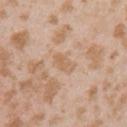<tbp_lesion>
  <biopsy_status>not biopsied; imaged during a skin examination</biopsy_status>
  <image>
    <source>total-body photography crop</source>
    <field_of_view_mm>15</field_of_view_mm>
  </image>
  <lighting>white-light</lighting>
  <patient>
    <sex>female</sex>
    <age_approx>25</age_approx>
  </patient>
  <lesion_size>
    <long_diameter_mm_approx>3.0</long_diameter_mm_approx>
  </lesion_size>
  <site>left upper arm</site>
</tbp_lesion>